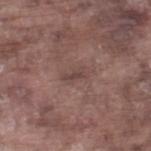Q: Was this lesion biopsied?
A: total-body-photography surveillance lesion; no biopsy
Q: Lesion location?
A: the right lower leg
Q: Patient demographics?
A: male, in their mid- to late 70s
Q: What kind of image is this?
A: ~15 mm crop, total-body skin-cancer survey
Q: What is the lesion's diameter?
A: about 2.5 mm
Q: What lighting was used for the tile?
A: white-light illumination
Q: What did automated image analysis measure?
A: a lesion color around L≈43 a*≈18 b*≈19 in CIELAB, roughly 7 lightness units darker than nearby skin, and a lesion-to-skin contrast of about 5.5 (normalized; higher = more distinct); a border-irregularity index near 5/10 and peripheral color asymmetry of about 0